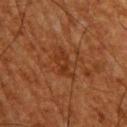This lesion was catalogued during total-body skin photography and was not selected for biopsy.
The lesion is located on the upper back.
Captured under cross-polarized illumination.
A male subject, about 65 years old.
Automated image analysis of the tile measured an average lesion color of about L≈28 a*≈21 b*≈30 (CIELAB) and a normalized lesion–skin contrast near 6. The software also gave a border-irregularity index near 5/10, a within-lesion color-variation index near 2/10, and peripheral color asymmetry of about 0.5.
Cropped from a total-body skin-imaging series; the visible field is about 15 mm.